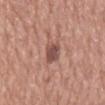Notes:
• follow-up · no biopsy performed (imaged during a skin exam)
• diameter · about 3 mm
• imaging modality · ~15 mm tile from a whole-body skin photo
• site · the mid back
• illumination · white-light illumination
• patient · male, roughly 75 years of age